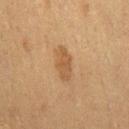<lesion>
<biopsy_status>not biopsied; imaged during a skin examination</biopsy_status>
</lesion>